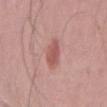biopsy status: total-body-photography surveillance lesion; no biopsy
patient: male, roughly 40 years of age
image source: 15 mm crop, total-body photography
location: the arm